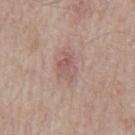Impression:
This lesion was catalogued during total-body skin photography and was not selected for biopsy.
Clinical summary:
A male subject, in their mid-60s. From the mid back. A 15 mm crop from a total-body photograph taken for skin-cancer surveillance. The recorded lesion diameter is about 3.5 mm. This is a white-light tile.